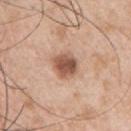This lesion was catalogued during total-body skin photography and was not selected for biopsy. Approximately 3.5 mm at its widest. A male subject aged around 55. On the right upper arm. A 15 mm crop from a total-body photograph taken for skin-cancer surveillance. The tile uses white-light illumination. The total-body-photography lesion software estimated an area of roughly 7.5 mm², a shape eccentricity near 0.55, and a shape-asymmetry score of about 0.2 (0 = symmetric). The analysis additionally found about 15 CIELAB-L* units darker than the surrounding skin and a lesion-to-skin contrast of about 10 (normalized; higher = more distinct). The analysis additionally found a color-variation rating of about 4/10 and peripheral color asymmetry of about 1.5. It also reported a nevus-likeness score of about 80/100 and a lesion-detection confidence of about 100/100.